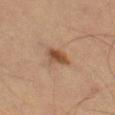{
  "biopsy_status": "not biopsied; imaged during a skin examination",
  "lighting": "cross-polarized",
  "lesion_size": {
    "long_diameter_mm_approx": 3.0
  },
  "automated_metrics": {
    "nevus_likeness_0_100": 85
  },
  "patient": {
    "sex": "male",
    "age_approx": 60
  },
  "site": "leg",
  "image": {
    "source": "total-body photography crop",
    "field_of_view_mm": 15
  }
}